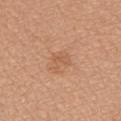* biopsy status: no biopsy performed (imaged during a skin exam)
* automated lesion analysis: a lesion area of about 4 mm² and a symmetry-axis asymmetry near 0.4; a border-irregularity index near 5/10, internal color variation of about 1.5 on a 0–10 scale, and a peripheral color-asymmetry measure near 0.5
* anatomic site: the lower back
* lighting: white-light illumination
* subject: male, aged 63 to 67
* lesion diameter: ≈2.5 mm
* image source: ~15 mm crop, total-body skin-cancer survey